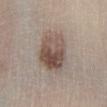This lesion was catalogued during total-body skin photography and was not selected for biopsy. A male subject, roughly 65 years of age. Located on the left lower leg. A close-up tile cropped from a whole-body skin photograph, about 15 mm across. The tile uses white-light illumination. The lesion's longest dimension is about 5 mm. An algorithmic analysis of the crop reported about 12 CIELAB-L* units darker than the surrounding skin and a lesion-to-skin contrast of about 9 (normalized; higher = more distinct). And it measured a border-irregularity rating of about 1.5/10, a color-variation rating of about 8/10, and peripheral color asymmetry of about 3.5.